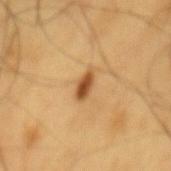workup: total-body-photography surveillance lesion; no biopsy
image: total-body-photography crop, ~15 mm field of view
lighting: cross-polarized illumination
patient: male, in their 60s
diameter: about 3 mm
location: the mid back